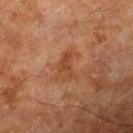Part of a total-body skin-imaging series; this lesion was reviewed on a skin check and was not flagged for biopsy. From the left thigh. The patient is a male in their 80s. A lesion tile, about 15 mm wide, cut from a 3D total-body photograph.